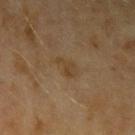Imaged during a routine full-body skin examination; the lesion was not biopsied and no histopathology is available. Cropped from a total-body skin-imaging series; the visible field is about 15 mm. A female patient in their 60s. Located on the left upper arm. The lesion-visualizer software estimated two-axis asymmetry of about 0.4. It also reported a lesion color around L≈37 a*≈13 b*≈29 in CIELAB and about 5 CIELAB-L* units darker than the surrounding skin. It also reported a border-irregularity rating of about 4.5/10, a color-variation rating of about 2/10, and a peripheral color-asymmetry measure near 0.5. It also reported a lesion-detection confidence of about 100/100. The tile uses cross-polarized illumination.